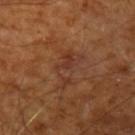<tbp_lesion>
<biopsy_status>not biopsied; imaged during a skin examination</biopsy_status>
<image>
  <source>total-body photography crop</source>
  <field_of_view_mm>15</field_of_view_mm>
</image>
<patient>
  <age_approx>65</age_approx>
</patient>
<lighting>cross-polarized</lighting>
<automated_metrics>
  <cielab_L>35</cielab_L>
  <cielab_a>22</cielab_a>
  <cielab_b>28</cielab_b>
  <vs_skin_darker_L>5.0</vs_skin_darker_L>
  <vs_skin_contrast_norm>5.0</vs_skin_contrast_norm>
  <nevus_likeness_0_100>0</nevus_likeness_0_100>
  <lesion_detection_confidence_0_100>100</lesion_detection_confidence_0_100>
</automated_metrics>
<site>arm</site>
</tbp_lesion>